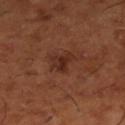follow-up = no biopsy performed (imaged during a skin exam)